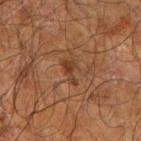Assessment:
No biopsy was performed on this lesion — it was imaged during a full skin examination and was not determined to be concerning.
Background:
This is a cross-polarized tile. The subject is a male about 70 years old. The lesion is located on the left forearm. Automated image analysis of the tile measured a border-irregularity index near 6/10, a within-lesion color-variation index near 1/10, and peripheral color asymmetry of about 0.5. The analysis additionally found a nevus-likeness score of about 0/100 and a lesion-detection confidence of about 100/100. A 15 mm crop from a total-body photograph taken for skin-cancer surveillance.